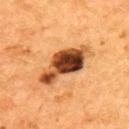Recorded during total-body skin imaging; not selected for excision or biopsy.
The patient is a male approximately 55 years of age.
From the back.
A close-up tile cropped from a whole-body skin photograph, about 15 mm across.